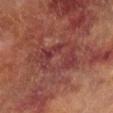Captured during whole-body skin photography for melanoma surveillance; the lesion was not biopsied.
On the right lower leg.
A region of skin cropped from a whole-body photographic capture, roughly 15 mm wide.
The patient is a male about 70 years old.
Measured at roughly 7.5 mm in maximum diameter.
The tile uses cross-polarized illumination.